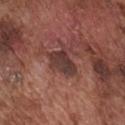follow-up = catalogued during a skin exam; not biopsied
lighting = white-light
body site = the chest
subject = male, in their mid- to late 70s
image source = 15 mm crop, total-body photography